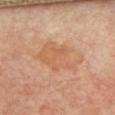Measured at roughly 5.5 mm in maximum diameter. A lesion tile, about 15 mm wide, cut from a 3D total-body photograph. A female subject, approximately 50 years of age. The lesion is located on the chest.